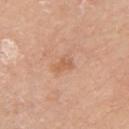notes — total-body-photography surveillance lesion; no biopsy
image source — ~15 mm tile from a whole-body skin photo
diameter — ≈2.5 mm
patient — male, roughly 80 years of age
tile lighting — white-light
location — the right upper arm
automated metrics — a footprint of about 3.5 mm² and a shape-asymmetry score of about 0.4 (0 = symmetric); a border-irregularity index near 3.5/10, a color-variation rating of about 1.5/10, and radial color variation of about 0.5